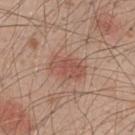Imaged during a routine full-body skin examination; the lesion was not biopsied and no histopathology is available.
The recorded lesion diameter is about 4 mm.
A male subject, aged 48–52.
The lesion-visualizer software estimated a lesion area of about 9 mm², an eccentricity of roughly 0.8, and a shape-asymmetry score of about 0.2 (0 = symmetric). The software also gave a lesion–skin lightness drop of about 8 and a normalized lesion–skin contrast near 6. The software also gave a border-irregularity rating of about 2/10 and peripheral color asymmetry of about 1. The software also gave a classifier nevus-likeness of about 75/100.
The lesion is located on the upper back.
This image is a 15 mm lesion crop taken from a total-body photograph.